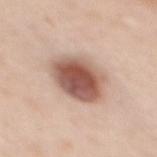Clinical impression: Recorded during total-body skin imaging; not selected for excision or biopsy. Clinical summary: From the back. The subject is a female approximately 50 years of age. Approximately 5 mm at its widest. A 15 mm close-up extracted from a 3D total-body photography capture. Captured under white-light illumination.